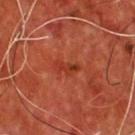Captured under cross-polarized illumination.
Cropped from a whole-body photographic skin survey; the tile spans about 15 mm.
The patient is a male aged approximately 55.
Located on the chest.
The lesion's longest dimension is about 3 mm.